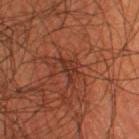notes: no biopsy performed (imaged during a skin exam)
location: the left lower leg
imaging modality: 15 mm crop, total-body photography
lighting: cross-polarized
TBP lesion metrics: a lesion color around L≈31 a*≈24 b*≈27 in CIELAB, roughly 7 lightness units darker than nearby skin, and a normalized border contrast of about 6.5; peripheral color asymmetry of about 0.5; an automated nevus-likeness rating near 0 out of 100
lesion diameter: ~3.5 mm (longest diameter)
patient: male, roughly 55 years of age